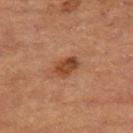The lesion was photographed on a routine skin check and not biopsied; there is no pathology result.
Cropped from a whole-body photographic skin survey; the tile spans about 15 mm.
Imaged with cross-polarized lighting.
The lesion is located on the right thigh.
The lesion's longest dimension is about 3 mm.
The patient is a female aged 58–62.